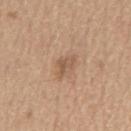Recorded during total-body skin imaging; not selected for excision or biopsy. The lesion is on the chest. Imaged with white-light lighting. A lesion tile, about 15 mm wide, cut from a 3D total-body photograph. A male patient roughly 70 years of age. An algorithmic analysis of the crop reported an area of roughly 3 mm² and a shape eccentricity near 0.8. The software also gave a mean CIELAB color near L≈55 a*≈18 b*≈31, roughly 9 lightness units darker than nearby skin, and a lesion-to-skin contrast of about 6 (normalized; higher = more distinct). It also reported a nevus-likeness score of about 5/100 and lesion-presence confidence of about 100/100. Approximately 3 mm at its widest.